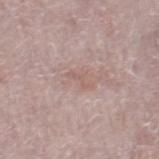{"biopsy_status": "not biopsied; imaged during a skin examination", "lesion_size": {"long_diameter_mm_approx": 3.0}, "site": "right thigh", "lighting": "white-light", "automated_metrics": {"area_mm2_approx": 2.0, "eccentricity": 0.95, "shape_asymmetry": 0.6, "cielab_L": 58, "cielab_a": 19, "cielab_b": 23, "vs_skin_contrast_norm": 5.0, "border_irregularity_0_10": 8.0, "color_variation_0_10": 0.0, "nevus_likeness_0_100": 0, "lesion_detection_confidence_0_100": 100}, "patient": {"sex": "female", "age_approx": 65}, "image": {"source": "total-body photography crop", "field_of_view_mm": 15}}